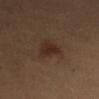Acquisition and patient details:
The patient is a female about 40 years old. Cropped from a whole-body photographic skin survey; the tile spans about 15 mm. From the left upper arm. Captured under cross-polarized illumination.